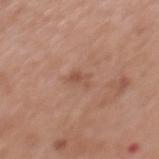biopsy status: catalogued during a skin exam; not biopsied | lesion diameter: about 2.5 mm | automated metrics: a lesion area of about 3 mm², an outline eccentricity of about 0.8 (0 = round, 1 = elongated), and a symmetry-axis asymmetry near 0.45; a mean CIELAB color near L≈52 a*≈21 b*≈29 and about 7 CIELAB-L* units darker than the surrounding skin; border irregularity of about 4 on a 0–10 scale, internal color variation of about 1 on a 0–10 scale, and a peripheral color-asymmetry measure near 0.5; a nevus-likeness score of about 0/100 | patient: male, about 70 years old | image source: total-body-photography crop, ~15 mm field of view | anatomic site: the mid back.